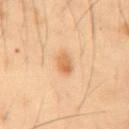Clinical summary:
Longest diameter approximately 2.5 mm. Automated image analysis of the tile measured a border-irregularity rating of about 1.5/10, a within-lesion color-variation index near 3/10, and a peripheral color-asymmetry measure near 1. From the abdomen. The patient is a male aged 53–57. A lesion tile, about 15 mm wide, cut from a 3D total-body photograph.